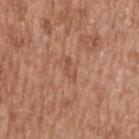Part of a total-body skin-imaging series; this lesion was reviewed on a skin check and was not flagged for biopsy. This is a white-light tile. The recorded lesion diameter is about 3 mm. On the upper back. The lesion-visualizer software estimated a border-irregularity index near 8/10, internal color variation of about 0 on a 0–10 scale, and radial color variation of about 0. And it measured an automated nevus-likeness rating near 0 out of 100 and a detector confidence of about 100 out of 100 that the crop contains a lesion. Cropped from a whole-body photographic skin survey; the tile spans about 15 mm. A male patient, aged 63 to 67.